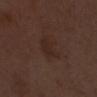{
  "biopsy_status": "not biopsied; imaged during a skin examination",
  "lesion_size": {
    "long_diameter_mm_approx": 3.5
  },
  "image": {
    "source": "total-body photography crop",
    "field_of_view_mm": 15
  },
  "lighting": "white-light",
  "patient": {
    "sex": "female",
    "age_approx": 50
  },
  "site": "chest"
}